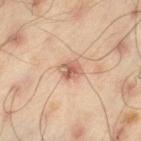* workup — total-body-photography surveillance lesion; no biopsy
* subject — male, aged 43 to 47
* lesion diameter — ≈2.5 mm
* acquisition — 15 mm crop, total-body photography
* body site — the left thigh